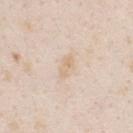– notes · catalogued during a skin exam; not biopsied
– image-analysis metrics · a lesion–skin lightness drop of about 6 and a lesion-to-skin contrast of about 5.5 (normalized; higher = more distinct)
– illumination · white-light
– lesion diameter · ≈3 mm
– patient · female, in their mid-20s
– site · the upper back
– imaging modality · total-body-photography crop, ~15 mm field of view